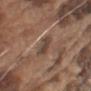The lesion was tiled from a total-body skin photograph and was not biopsied. Automated tile analysis of the lesion measured an average lesion color of about L≈43 a*≈16 b*≈24 (CIELAB), about 9 CIELAB-L* units darker than the surrounding skin, and a normalized lesion–skin contrast near 7.5. And it measured a border-irregularity index near 3/10 and peripheral color asymmetry of about 1. The software also gave an automated nevus-likeness rating near 5 out of 100 and a detector confidence of about 95 out of 100 that the crop contains a lesion. This image is a 15 mm lesion crop taken from a total-body photograph. From the left upper arm. The subject is a male roughly 75 years of age. Imaged with white-light lighting. Approximately 2.5 mm at its widest.